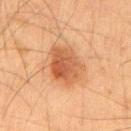<tbp_lesion>
  <biopsy_status>not biopsied; imaged during a skin examination</biopsy_status>
  <patient>
    <sex>male</sex>
    <age_approx>35</age_approx>
  </patient>
  <lesion_size>
    <long_diameter_mm_approx>5.5</long_diameter_mm_approx>
  </lesion_size>
  <lighting>cross-polarized</lighting>
  <automated_metrics>
    <cielab_L>45</cielab_L>
    <cielab_a>21</cielab_a>
    <cielab_b>32</cielab_b>
    <vs_skin_darker_L>10.0</vs_skin_darker_L>
    <vs_skin_contrast_norm>7.5</vs_skin_contrast_norm>
  </automated_metrics>
  <site>mid back</site>
  <image>
    <source>total-body photography crop</source>
    <field_of_view_mm>15</field_of_view_mm>
  </image>
</tbp_lesion>